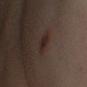Imaged during a routine full-body skin examination; the lesion was not biopsied and no histopathology is available. A 15 mm close-up tile from a total-body photography series done for melanoma screening. A male patient, aged around 35. From the left leg. Measured at roughly 3 mm in maximum diameter. Captured under cross-polarized illumination.